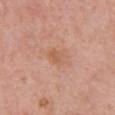<tbp_lesion>
  <biopsy_status>not biopsied; imaged during a skin examination</biopsy_status>
  <image>
    <source>total-body photography crop</source>
    <field_of_view_mm>15</field_of_view_mm>
  </image>
  <site>chest</site>
  <patient>
    <sex>female</sex>
    <age_approx>40</age_approx>
  </patient>
  <automated_metrics>
    <area_mm2_approx>4.5</area_mm2_approx>
    <shape_asymmetry>0.2</shape_asymmetry>
    <cielab_L>59</cielab_L>
    <cielab_a>22</cielab_a>
    <cielab_b>33</cielab_b>
    <vs_skin_darker_L>6.0</vs_skin_darker_L>
    <vs_skin_contrast_norm>5.5</vs_skin_contrast_norm>
  </automated_metrics>
</tbp_lesion>